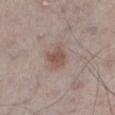workup — imaged on a skin check; not biopsied | TBP lesion metrics — an area of roughly 6 mm², an eccentricity of roughly 0.45, and a shape-asymmetry score of about 0.25 (0 = symmetric); a mean CIELAB color near L≈52 a*≈17 b*≈23, a lesion–skin lightness drop of about 9, and a normalized border contrast of about 7; an automated nevus-likeness rating near 90 out of 100 and a detector confidence of about 100 out of 100 that the crop contains a lesion | imaging modality — total-body-photography crop, ~15 mm field of view | subject — male, in their 60s | site — the left lower leg.